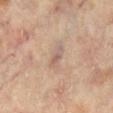lighting: cross-polarized
image:
  source: total-body photography crop
  field_of_view_mm: 15
lesion_size:
  long_diameter_mm_approx: 2.5
patient:
  sex: female
  age_approx: 65
automated_metrics:
  area_mm2_approx: 2.5
  eccentricity: 0.9
  cielab_L: 57
  cielab_a: 16
  cielab_b: 24
  vs_skin_contrast_norm: 6.0
  border_irregularity_0_10: 3.0
  color_variation_0_10: 0.0
  nevus_likeness_0_100: 0
  lesion_detection_confidence_0_100: 95
site: left leg